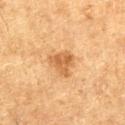The lesion was tiled from a total-body skin photograph and was not biopsied. This is a cross-polarized tile. Cropped from a total-body skin-imaging series; the visible field is about 15 mm. Automated image analysis of the tile measured a mean CIELAB color near L≈49 a*≈20 b*≈36 and a lesion-to-skin contrast of about 7 (normalized; higher = more distinct). The software also gave internal color variation of about 3 on a 0–10 scale. It also reported a classifier nevus-likeness of about 70/100 and a lesion-detection confidence of about 100/100. Measured at roughly 3.5 mm in maximum diameter. The patient is a male aged around 75. Located on the leg.